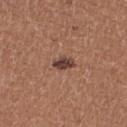| key | value |
|---|---|
| image | ~15 mm tile from a whole-body skin photo |
| image-analysis metrics | a lesion color around L≈41 a*≈20 b*≈24 in CIELAB and a normalized border contrast of about 11; a classifier nevus-likeness of about 55/100 |
| body site | the right thigh |
| subject | female, about 35 years old |